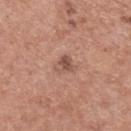Q: Is there a histopathology result?
A: imaged on a skin check; not biopsied
Q: What is the anatomic site?
A: the upper back
Q: What are the patient's age and sex?
A: female, aged around 60
Q: Automated lesion metrics?
A: a lesion color around L≈52 a*≈22 b*≈26 in CIELAB, roughly 10 lightness units darker than nearby skin, and a normalized border contrast of about 7; internal color variation of about 4.5 on a 0–10 scale; a nevus-likeness score of about 65/100 and a lesion-detection confidence of about 100/100
Q: What is the lesion's diameter?
A: ≈2.5 mm
Q: Illumination type?
A: white-light illumination
Q: How was this image acquired?
A: total-body-photography crop, ~15 mm field of view